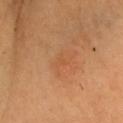No biopsy was performed on this lesion — it was imaged during a full skin examination and was not determined to be concerning.